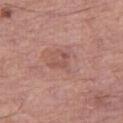{"biopsy_status": "not biopsied; imaged during a skin examination", "lesion_size": {"long_diameter_mm_approx": 2.5}, "lighting": "white-light", "image": {"source": "total-body photography crop", "field_of_view_mm": 15}, "automated_metrics": {"vs_skin_darker_L": 7.0, "vs_skin_contrast_norm": 5.0, "border_irregularity_0_10": 8.0, "color_variation_0_10": 0.0, "peripheral_color_asymmetry": 0.0}, "patient": {"sex": "male", "age_approx": 65}, "site": "right thigh"}